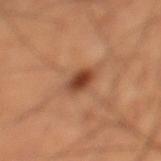Imaged during a routine full-body skin examination; the lesion was not biopsied and no histopathology is available.
Automated tile analysis of the lesion measured a lesion–skin lightness drop of about 14 and a lesion-to-skin contrast of about 10 (normalized; higher = more distinct). And it measured a border-irregularity rating of about 2/10 and a peripheral color-asymmetry measure near 1.5.
The lesion is on the left lower leg.
A 15 mm close-up tile from a total-body photography series done for melanoma screening.
Imaged with cross-polarized lighting.
A male subject approximately 60 years of age.
About 3 mm across.